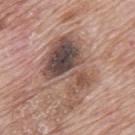{
  "biopsy_status": "not biopsied; imaged during a skin examination",
  "site": "mid back",
  "image": {
    "source": "total-body photography crop",
    "field_of_view_mm": 15
  },
  "patient": {
    "sex": "male",
    "age_approx": 70
  }
}